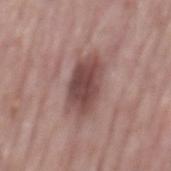Case summary:
– notes — imaged on a skin check; not biopsied
– imaging modality — 15 mm crop, total-body photography
– patient — male, about 65 years old
– body site — the mid back